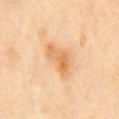automated metrics: a shape eccentricity near 0.7 and a symmetry-axis asymmetry near 0.45; an average lesion color of about L≈71 a*≈23 b*≈44 (CIELAB), a lesion–skin lightness drop of about 10, and a lesion-to-skin contrast of about 6.5 (normalized; higher = more distinct)
image: ~15 mm crop, total-body skin-cancer survey
anatomic site: the front of the torso